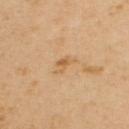The lesion was photographed on a routine skin check and not biopsied; there is no pathology result. The tile uses cross-polarized illumination. The lesion is located on the upper back. A lesion tile, about 15 mm wide, cut from a 3D total-body photograph. A male subject, roughly 55 years of age. The lesion's longest dimension is about 2.5 mm. Automated tile analysis of the lesion measured a lesion color around L≈62 a*≈19 b*≈41 in CIELAB and roughly 8 lightness units darker than nearby skin. The analysis additionally found border irregularity of about 4.5 on a 0–10 scale, a within-lesion color-variation index near 2/10, and radial color variation of about 0.5. It also reported a classifier nevus-likeness of about 0/100.